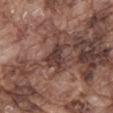Q: Is there a histopathology result?
A: catalogued during a skin exam; not biopsied